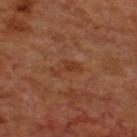This lesion was catalogued during total-body skin photography and was not selected for biopsy. A close-up tile cropped from a whole-body skin photograph, about 15 mm across. A male patient, aged around 70. The lesion's longest dimension is about 3.5 mm. The lesion is on the upper back. The tile uses cross-polarized illumination.